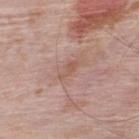Q: Is there a histopathology result?
A: total-body-photography surveillance lesion; no biopsy
Q: Illumination type?
A: white-light illumination
Q: What kind of image is this?
A: ~15 mm crop, total-body skin-cancer survey
Q: What are the patient's age and sex?
A: male, about 50 years old
Q: Lesion location?
A: the upper back
Q: What did automated image analysis measure?
A: an average lesion color of about L≈55 a*≈20 b*≈27 (CIELAB) and a lesion–skin lightness drop of about 7; a border-irregularity index near 5/10, internal color variation of about 0 on a 0–10 scale, and a peripheral color-asymmetry measure near 0; lesion-presence confidence of about 100/100